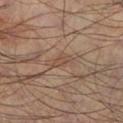Clinical impression:
Part of a total-body skin-imaging series; this lesion was reviewed on a skin check and was not flagged for biopsy.
Image and clinical context:
The tile uses cross-polarized illumination. The lesion's longest dimension is about 3 mm. A region of skin cropped from a whole-body photographic capture, roughly 15 mm wide. A male subject approximately 55 years of age. Located on the left lower leg.